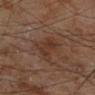{
  "biopsy_status": "not biopsied; imaged during a skin examination",
  "patient": {
    "sex": "male",
    "age_approx": 70
  },
  "automated_metrics": {
    "area_mm2_approx": 8.5,
    "eccentricity": 0.85,
    "shape_asymmetry": 0.45,
    "border_irregularity_0_10": 5.0,
    "peripheral_color_asymmetry": 1.0,
    "nevus_likeness_0_100": 5,
    "lesion_detection_confidence_0_100": 100
  },
  "site": "left lower leg",
  "lesion_size": {
    "long_diameter_mm_approx": 4.5
  },
  "image": {
    "source": "total-body photography crop",
    "field_of_view_mm": 15
  },
  "lighting": "cross-polarized"
}